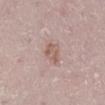{
  "biopsy_status": "not biopsied; imaged during a skin examination",
  "patient": {
    "sex": "female",
    "age_approx": 25
  },
  "image": {
    "source": "total-body photography crop",
    "field_of_view_mm": 15
  },
  "site": "leg",
  "lighting": "white-light",
  "lesion_size": {
    "long_diameter_mm_approx": 2.5
  }
}